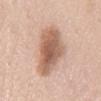The lesion was tiled from a total-body skin photograph and was not biopsied.